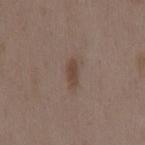Q: Was a biopsy performed?
A: no biopsy performed (imaged during a skin exam)
Q: Lesion location?
A: the mid back
Q: Automated lesion metrics?
A: a lesion color around L≈43 a*≈16 b*≈23 in CIELAB and a lesion–skin lightness drop of about 9; border irregularity of about 2.5 on a 0–10 scale, internal color variation of about 0.5 on a 0–10 scale, and peripheral color asymmetry of about 0
Q: What is the lesion's diameter?
A: ≈2.5 mm
Q: What kind of image is this?
A: 15 mm crop, total-body photography
Q: Patient demographics?
A: female, aged approximately 35
Q: How was the tile lit?
A: white-light illumination